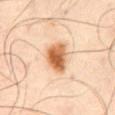| field | value |
|---|---|
| follow-up | no biopsy performed (imaged during a skin exam) |
| illumination | cross-polarized |
| body site | the abdomen |
| lesion diameter | about 4 mm |
| image source | ~15 mm tile from a whole-body skin photo |
| subject | male, in their mid- to late 60s |